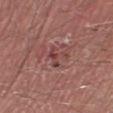A male patient about 55 years old. About 3 mm across. A lesion tile, about 15 mm wide, cut from a 3D total-body photograph. On the right lower leg. This is a white-light tile.